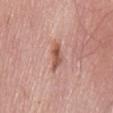Clinical impression: Recorded during total-body skin imaging; not selected for excision or biopsy. Acquisition and patient details: This is a white-light tile. Located on the mid back. A roughly 15 mm field-of-view crop from a total-body skin photograph. The lesion-visualizer software estimated a border-irregularity rating of about 4.5/10 and radial color variation of about 1. A male subject, aged approximately 70. The lesion's longest dimension is about 4 mm.